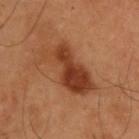Recorded during total-body skin imaging; not selected for excision or biopsy. Cropped from a total-body skin-imaging series; the visible field is about 15 mm. The patient is a male in their mid- to late 50s. The recorded lesion diameter is about 7 mm. Located on the back.